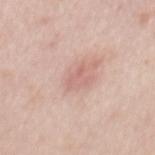Imaged during a routine full-body skin examination; the lesion was not biopsied and no histopathology is available. A roughly 15 mm field-of-view crop from a total-body skin photograph. The lesion is on the mid back. The total-body-photography lesion software estimated a shape eccentricity near 0.75 and a shape-asymmetry score of about 0.65 (0 = symmetric). The analysis additionally found a border-irregularity rating of about 8.5/10, a within-lesion color-variation index near 0/10, and peripheral color asymmetry of about 0. A male subject, approximately 40 years of age. Longest diameter approximately 3 mm.